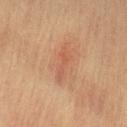Assessment: The lesion was photographed on a routine skin check and not biopsied; there is no pathology result. Image and clinical context: Captured under cross-polarized illumination. A 15 mm close-up extracted from a 3D total-body photography capture. The patient is a female in their mid-60s. Automated tile analysis of the lesion measured an area of roughly 5 mm², a shape eccentricity near 0.95, and two-axis asymmetry of about 0.4. It also reported a mean CIELAB color near L≈48 a*≈21 b*≈29, about 6 CIELAB-L* units darker than the surrounding skin, and a normalized lesion–skin contrast near 4.5. The software also gave a border-irregularity rating of about 5.5/10, a color-variation rating of about 1/10, and peripheral color asymmetry of about 0.5. On the leg.